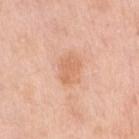Context:
From the right upper arm. Imaged with white-light lighting. The patient is a female aged 48–52. A 15 mm close-up tile from a total-body photography series done for melanoma screening.